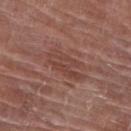Case summary:
- biopsy status — no biopsy performed (imaged during a skin exam)
- subject — female, aged 78–82
- lesion diameter — about 5 mm
- location — the right lower leg
- lighting — white-light illumination
- image — ~15 mm tile from a whole-body skin photo